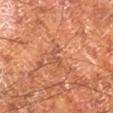Q: Is there a histopathology result?
A: imaged on a skin check; not biopsied
Q: Who is the patient?
A: male, aged approximately 65
Q: How was this image acquired?
A: 15 mm crop, total-body photography
Q: Automated lesion metrics?
A: an area of roughly 3 mm², an outline eccentricity of about 0.85 (0 = round, 1 = elongated), and a shape-asymmetry score of about 0.55 (0 = symmetric); internal color variation of about 0 on a 0–10 scale; a classifier nevus-likeness of about 0/100 and a detector confidence of about 85 out of 100 that the crop contains a lesion
Q: Lesion location?
A: the right lower leg
Q: Illumination type?
A: cross-polarized